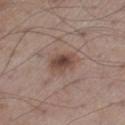The lesion was photographed on a routine skin check and not biopsied; there is no pathology result.
A lesion tile, about 15 mm wide, cut from a 3D total-body photograph.
A male subject roughly 55 years of age.
The lesion is on the left thigh.